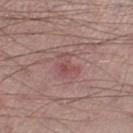A male patient in their mid- to late 50s.
This is a white-light tile.
On the right lower leg.
A roughly 15 mm field-of-view crop from a total-body skin photograph.
The lesion's longest dimension is about 3.5 mm.